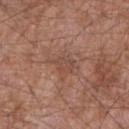<tbp_lesion>
<biopsy_status>not biopsied; imaged during a skin examination</biopsy_status>
<patient>
  <sex>male</sex>
  <age_approx>55</age_approx>
</patient>
<lighting>white-light</lighting>
<image>
  <source>total-body photography crop</source>
  <field_of_view_mm>15</field_of_view_mm>
</image>
<site>right forearm</site>
</tbp_lesion>